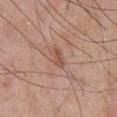Clinical impression: This lesion was catalogued during total-body skin photography and was not selected for biopsy. Background: A male patient, aged 58 to 62. The lesion is on the chest. A roughly 15 mm field-of-view crop from a total-body skin photograph.